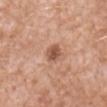Captured during whole-body skin photography for melanoma surveillance; the lesion was not biopsied.
Located on the abdomen.
Cropped from a whole-body photographic skin survey; the tile spans about 15 mm.
The patient is a male about 60 years old.